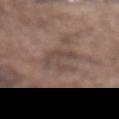The lesion was tiled from a total-body skin photograph and was not biopsied.
Located on the abdomen.
A male subject, roughly 75 years of age.
Automated image analysis of the tile measured roughly 7 lightness units darker than nearby skin. And it measured a border-irregularity index near 7.5/10 and a color-variation rating of about 2/10. The software also gave a classifier nevus-likeness of about 0/100 and a detector confidence of about 90 out of 100 that the crop contains a lesion.
Imaged with white-light lighting.
A roughly 15 mm field-of-view crop from a total-body skin photograph.